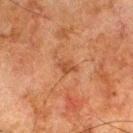The lesion was tiled from a total-body skin photograph and was not biopsied. A male patient, about 80 years old. Measured at roughly 2.5 mm in maximum diameter. The lesion is located on the leg. A 15 mm close-up extracted from a 3D total-body photography capture. Imaged with cross-polarized lighting. Automated tile analysis of the lesion measured an outline eccentricity of about 0.8 (0 = round, 1 = elongated) and a symmetry-axis asymmetry near 0.3. The analysis additionally found a lesion-to-skin contrast of about 6 (normalized; higher = more distinct). The analysis additionally found an automated nevus-likeness rating near 0 out of 100 and lesion-presence confidence of about 100/100.